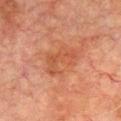Clinical impression: This lesion was catalogued during total-body skin photography and was not selected for biopsy. Context: A male patient approximately 75 years of age. An algorithmic analysis of the crop reported a lesion color around L≈44 a*≈25 b*≈31 in CIELAB, roughly 6 lightness units darker than nearby skin, and a normalized border contrast of about 5.5. The analysis additionally found a classifier nevus-likeness of about 0/100 and a lesion-detection confidence of about 100/100. Longest diameter approximately 5 mm. On the chest. This is a cross-polarized tile. A 15 mm close-up tile from a total-body photography series done for melanoma screening.